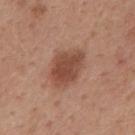Part of a total-body skin-imaging series; this lesion was reviewed on a skin check and was not flagged for biopsy. The tile uses white-light illumination. This image is a 15 mm lesion crop taken from a total-body photograph. Automated tile analysis of the lesion measured an outline eccentricity of about 0.7 (0 = round, 1 = elongated) and two-axis asymmetry of about 0.2. The analysis additionally found roughly 11 lightness units darker than nearby skin. The analysis additionally found a border-irregularity index near 2/10 and a within-lesion color-variation index near 3/10. The analysis additionally found a classifier nevus-likeness of about 85/100 and lesion-presence confidence of about 100/100. A male patient, about 50 years old. Located on the mid back.